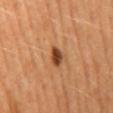biopsy_status: not biopsied; imaged during a skin examination
image:
  source: total-body photography crop
  field_of_view_mm: 15
lighting: cross-polarized
automated_metrics:
  cielab_L: 44
  cielab_a: 26
  cielab_b: 36
  vs_skin_darker_L: 16.0
  vs_skin_contrast_norm: 11.5
  nevus_likeness_0_100: 95
site: back
patient:
  sex: female
lesion_size:
  long_diameter_mm_approx: 2.5